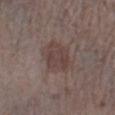follow-up = no biopsy performed (imaged during a skin exam) | subject = male, aged around 70 | image = ~15 mm crop, total-body skin-cancer survey | location = the right lower leg | illumination = white-light.